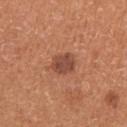Q: Is there a histopathology result?
A: total-body-photography surveillance lesion; no biopsy
Q: What did automated image analysis measure?
A: an area of roughly 5.5 mm², a shape eccentricity near 0.55, and a shape-asymmetry score of about 0.2 (0 = symmetric); a lesion color around L≈47 a*≈25 b*≈30 in CIELAB, a lesion–skin lightness drop of about 11, and a normalized lesion–skin contrast near 8; a classifier nevus-likeness of about 70/100 and a lesion-detection confidence of about 100/100
Q: Where on the body is the lesion?
A: the arm
Q: What is the imaging modality?
A: ~15 mm tile from a whole-body skin photo
Q: What lighting was used for the tile?
A: white-light
Q: Who is the patient?
A: female, about 35 years old